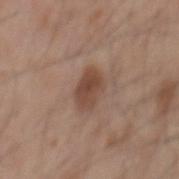<lesion>
<biopsy_status>not biopsied; imaged during a skin examination</biopsy_status>
<lighting>white-light</lighting>
<automated_metrics>
  <vs_skin_darker_L>10.0</vs_skin_darker_L>
  <vs_skin_contrast_norm>8.0</vs_skin_contrast_norm>
</automated_metrics>
<lesion_size>
  <long_diameter_mm_approx>4.0</long_diameter_mm_approx>
</lesion_size>
<patient>
  <sex>male</sex>
  <age_approx>55</age_approx>
</patient>
<site>back</site>
<image>
  <source>total-body photography crop</source>
  <field_of_view_mm>15</field_of_view_mm>
</image>
</lesion>